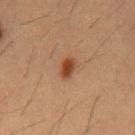The lesion was photographed on a routine skin check and not biopsied; there is no pathology result.
An algorithmic analysis of the crop reported an area of roughly 4 mm², an outline eccentricity of about 0.8 (0 = round, 1 = elongated), and a shape-asymmetry score of about 0.15 (0 = symmetric). It also reported an average lesion color of about L≈39 a*≈20 b*≈31 (CIELAB), about 10 CIELAB-L* units darker than the surrounding skin, and a lesion-to-skin contrast of about 9.5 (normalized; higher = more distinct). The analysis additionally found a within-lesion color-variation index near 3/10 and radial color variation of about 1. And it measured an automated nevus-likeness rating near 95 out of 100 and a lesion-detection confidence of about 100/100.
The tile uses cross-polarized illumination.
A male subject, aged 53–57.
Cropped from a whole-body photographic skin survey; the tile spans about 15 mm.
The lesion is on the chest.